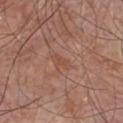Part of a total-body skin-imaging series; this lesion was reviewed on a skin check and was not flagged for biopsy. The tile uses white-light illumination. The recorded lesion diameter is about 3 mm. The lesion is located on the chest. This image is a 15 mm lesion crop taken from a total-body photograph. The subject is a male roughly 65 years of age.